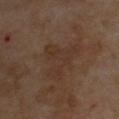Part of a total-body skin-imaging series; this lesion was reviewed on a skin check and was not flagged for biopsy. From the chest. The subject is a male in their 60s. Captured under cross-polarized illumination. A 15 mm crop from a total-body photograph taken for skin-cancer surveillance.